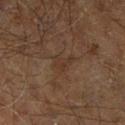No biopsy was performed on this lesion — it was imaged during a full skin examination and was not determined to be concerning.
A male patient, roughly 60 years of age.
The tile uses cross-polarized illumination.
From the leg.
About 2.5 mm across.
Cropped from a whole-body photographic skin survey; the tile spans about 15 mm.
Automated tile analysis of the lesion measured a shape eccentricity near 0.8 and a symmetry-axis asymmetry near 0.4.